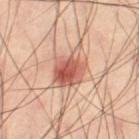The lesion was photographed on a routine skin check and not biopsied; there is no pathology result.